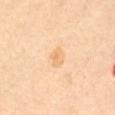| feature | finding |
|---|---|
| workup | no biopsy performed (imaged during a skin exam) |
| anatomic site | the mid back |
| lighting | cross-polarized illumination |
| subject | female, in their mid-70s |
| acquisition | ~15 mm tile from a whole-body skin photo |